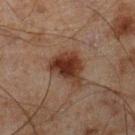follow-up=imaged on a skin check; not biopsied | lesion diameter=≈4.5 mm | location=the right lower leg | subject=male, about 45 years old | illumination=cross-polarized | image source=total-body-photography crop, ~15 mm field of view.